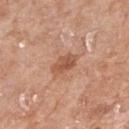Acquisition and patient details:
A region of skin cropped from a whole-body photographic capture, roughly 15 mm wide. A female subject in their mid- to late 70s. From the right forearm.